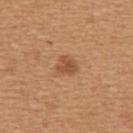No biopsy was performed on this lesion — it was imaged during a full skin examination and was not determined to be concerning.
Located on the upper back.
A female subject aged approximately 45.
Cropped from a total-body skin-imaging series; the visible field is about 15 mm.